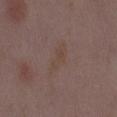– biopsy status — no biopsy performed (imaged during a skin exam)
– subject — female, roughly 35 years of age
– acquisition — 15 mm crop, total-body photography
– anatomic site — the lower back